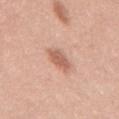Assessment: This lesion was catalogued during total-body skin photography and was not selected for biopsy. Context: A close-up tile cropped from a whole-body skin photograph, about 15 mm across. Imaged with white-light lighting. Located on the mid back. A male subject roughly 45 years of age. The lesion-visualizer software estimated a border-irregularity rating of about 3/10 and a within-lesion color-variation index near 2/10. It also reported a nevus-likeness score of about 80/100 and a lesion-detection confidence of about 100/100. Approximately 3 mm at its widest.